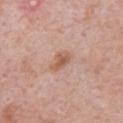Recorded during total-body skin imaging; not selected for excision or biopsy.